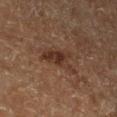biopsy_status: not biopsied; imaged during a skin examination
patient:
  sex: male
  age_approx: 75
image:
  source: total-body photography crop
  field_of_view_mm: 15
site: left lower leg